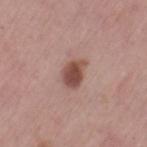Captured under white-light illumination. The recorded lesion diameter is about 3.5 mm. Located on the left thigh. A 15 mm close-up tile from a total-body photography series done for melanoma screening. The patient is a female in their mid- to late 60s.